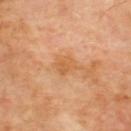biopsy status=no biopsy performed (imaged during a skin exam); acquisition=15 mm crop, total-body photography; site=the upper back; subject=male, aged 68 to 72; lighting=cross-polarized illumination.